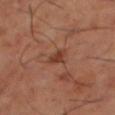{"biopsy_status": "not biopsied; imaged during a skin examination", "lighting": "cross-polarized", "site": "right thigh", "lesion_size": {"long_diameter_mm_approx": 2.5}, "image": {"source": "total-body photography crop", "field_of_view_mm": 15}, "patient": {"sex": "male", "age_approx": 65}}